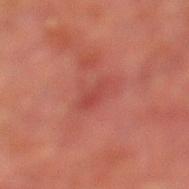Findings:
* notes · no biopsy performed (imaged during a skin exam)
* acquisition · ~15 mm crop, total-body skin-cancer survey
* automated metrics · an area of roughly 4.5 mm², an outline eccentricity of about 0.85 (0 = round, 1 = elongated), and a shape-asymmetry score of about 0.25 (0 = symmetric); a lesion color around L≈38 a*≈28 b*≈24 in CIELAB, roughly 5 lightness units darker than nearby skin, and a lesion-to-skin contrast of about 4.5 (normalized; higher = more distinct); a border-irregularity rating of about 3/10, internal color variation of about 2 on a 0–10 scale, and a peripheral color-asymmetry measure near 0.5; a nevus-likeness score of about 0/100 and a detector confidence of about 100 out of 100 that the crop contains a lesion
* patient · male, aged approximately 70
* anatomic site · the leg
* tile lighting · cross-polarized illumination